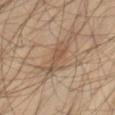Assessment: The lesion was tiled from a total-body skin photograph and was not biopsied. Clinical summary: A male patient aged approximately 45. The lesion-visualizer software estimated an area of roughly 5.5 mm², a shape eccentricity near 0.95, and two-axis asymmetry of about 0.4. The tile uses cross-polarized illumination. Cropped from a whole-body photographic skin survey; the tile spans about 15 mm. Located on the right thigh.